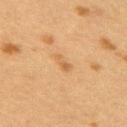Findings:
- biopsy status · total-body-photography surveillance lesion; no biopsy
- size · ~2.5 mm (longest diameter)
- automated metrics · a footprint of about 3 mm², a shape eccentricity near 0.85, and two-axis asymmetry of about 0.2; a border-irregularity rating of about 2.5/10 and internal color variation of about 0.5 on a 0–10 scale; a classifier nevus-likeness of about 40/100 and a lesion-detection confidence of about 100/100
- tile lighting · cross-polarized
- imaging modality · ~15 mm tile from a whole-body skin photo
- site · the right upper arm
- subject · female, aged around 40